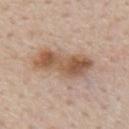Q: Is there a histopathology result?
A: catalogued during a skin exam; not biopsied
Q: Lesion size?
A: about 7 mm
Q: Patient demographics?
A: male, aged approximately 60
Q: Lesion location?
A: the mid back
Q: What is the imaging modality?
A: ~15 mm tile from a whole-body skin photo
Q: What lighting was used for the tile?
A: white-light illumination